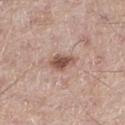Q: Illumination type?
A: white-light illumination
Q: What is the imaging modality?
A: ~15 mm tile from a whole-body skin photo
Q: How large is the lesion?
A: ~3 mm (longest diameter)
Q: Lesion location?
A: the right thigh
Q: Who is the patient?
A: male, aged approximately 65